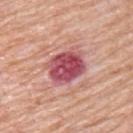{"biopsy_status": "not biopsied; imaged during a skin examination", "image": {"source": "total-body photography crop", "field_of_view_mm": 15}, "lighting": "white-light", "patient": {"sex": "male", "age_approx": 80}, "site": "left upper arm", "automated_metrics": {"area_mm2_approx": 15.0, "eccentricity": 0.6, "shape_asymmetry": 0.15, "cielab_L": 52, "cielab_a": 33, "cielab_b": 20, "vs_skin_darker_L": 16.0, "border_irregularity_0_10": 2.0, "color_variation_0_10": 7.0, "peripheral_color_asymmetry": 2.0, "nevus_likeness_0_100": 0}}